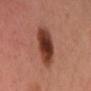Q: Was this lesion biopsied?
A: total-body-photography surveillance lesion; no biopsy
Q: Patient demographics?
A: female, aged around 40
Q: Lesion location?
A: the front of the torso
Q: How was this image acquired?
A: 15 mm crop, total-body photography